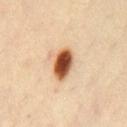notes = total-body-photography surveillance lesion; no biopsy.